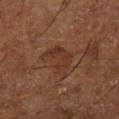{
  "biopsy_status": "not biopsied; imaged during a skin examination",
  "site": "left lower leg",
  "patient": {
    "sex": "male",
    "age_approx": 65
  },
  "image": {
    "source": "total-body photography crop",
    "field_of_view_mm": 15
  }
}